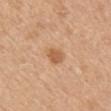Assessment:
No biopsy was performed on this lesion — it was imaged during a full skin examination and was not determined to be concerning.
Context:
The lesion is located on the back. A 15 mm crop from a total-body photograph taken for skin-cancer surveillance. A female subject, roughly 55 years of age.